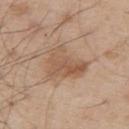The lesion was photographed on a routine skin check and not biopsied; there is no pathology result. This is a white-light tile. This image is a 15 mm lesion crop taken from a total-body photograph. On the upper back. A male patient about 55 years old.